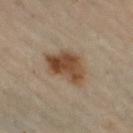No biopsy was performed on this lesion — it was imaged during a full skin examination and was not determined to be concerning. Longest diameter approximately 5 mm. A lesion tile, about 15 mm wide, cut from a 3D total-body photograph. The lesion-visualizer software estimated a lesion color around L≈46 a*≈16 b*≈30 in CIELAB, roughly 12 lightness units darker than nearby skin, and a normalized border contrast of about 10. It also reported an automated nevus-likeness rating near 95 out of 100 and a lesion-detection confidence of about 100/100. From the right thigh. Imaged with cross-polarized lighting. A female patient aged 58–62.